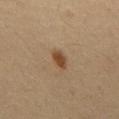Impression:
Imaged during a routine full-body skin examination; the lesion was not biopsied and no histopathology is available.
Image and clinical context:
The patient is a male in their mid-60s. Cropped from a total-body skin-imaging series; the visible field is about 15 mm. On the mid back.